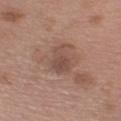- workup: catalogued during a skin exam; not biopsied
- acquisition: ~15 mm tile from a whole-body skin photo
- lighting: white-light
- automated lesion analysis: a lesion area of about 7 mm² and an eccentricity of roughly 0.75; a nevus-likeness score of about 0/100 and lesion-presence confidence of about 100/100
- location: the right upper arm
- subject: male, roughly 55 years of age